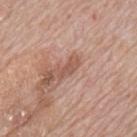{
  "biopsy_status": "not biopsied; imaged during a skin examination",
  "lighting": "white-light",
  "image": {
    "source": "total-body photography crop",
    "field_of_view_mm": 15
  },
  "patient": {
    "sex": "male",
    "age_approx": 70
  },
  "site": "mid back"
}